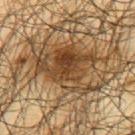follow-up: imaged on a skin check; not biopsied | anatomic site: the upper back | patient: male, in their mid- to late 60s | automated lesion analysis: a mean CIELAB color near L≈42 a*≈16 b*≈33, about 12 CIELAB-L* units darker than the surrounding skin, and a lesion-to-skin contrast of about 10 (normalized; higher = more distinct); a border-irregularity index near 6.5/10 and a within-lesion color-variation index near 7.5/10 | lighting: cross-polarized illumination | image source: 15 mm crop, total-body photography | lesion diameter: ≈8.5 mm.